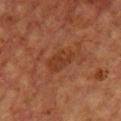No biopsy was performed on this lesion — it was imaged during a full skin examination and was not determined to be concerning. The lesion is located on the chest. About 3.5 mm across. A male patient, approximately 60 years of age. A roughly 15 mm field-of-view crop from a total-body skin photograph. This is a cross-polarized tile.